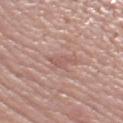Q: Is there a histopathology result?
A: no biopsy performed (imaged during a skin exam)
Q: Where on the body is the lesion?
A: the right thigh
Q: What did automated image analysis measure?
A: two-axis asymmetry of about 0.5; a lesion color around L≈56 a*≈22 b*≈24 in CIELAB, roughly 7 lightness units darker than nearby skin, and a normalized lesion–skin contrast near 5; a border-irregularity index near 5/10 and peripheral color asymmetry of about 0.5
Q: Who is the patient?
A: female, in their 40s
Q: What is the lesion's diameter?
A: about 3 mm
Q: What is the imaging modality?
A: 15 mm crop, total-body photography